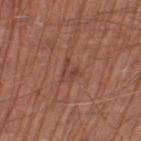biopsy_status: not biopsied; imaged during a skin examination
site: left thigh
automated_metrics:
  cielab_L: 43
  cielab_a: 23
  cielab_b: 27
  vs_skin_darker_L: 7.0
  vs_skin_contrast_norm: 5.5
  border_irregularity_0_10: 8.0
  color_variation_0_10: 0.0
  nevus_likeness_0_100: 0
lesion_size:
  long_diameter_mm_approx: 2.5
patient:
  sex: male
  age_approx: 65
image:
  source: total-body photography crop
  field_of_view_mm: 15